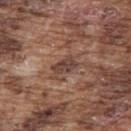The lesion was tiled from a total-body skin photograph and was not biopsied.
Located on the upper back.
The patient is a male aged approximately 75.
This is a white-light tile.
A 15 mm crop from a total-body photograph taken for skin-cancer surveillance.